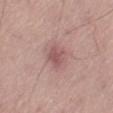The lesion was tiled from a total-body skin photograph and was not biopsied. Approximately 3 mm at its widest. Automated tile analysis of the lesion measured an outline eccentricity of about 0.6 (0 = round, 1 = elongated) and a symmetry-axis asymmetry near 0.25. It also reported a nevus-likeness score of about 0/100 and lesion-presence confidence of about 100/100. Captured under white-light illumination. A 15 mm close-up tile from a total-body photography series done for melanoma screening. A male subject aged approximately 75. The lesion is on the leg.